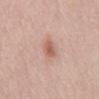workup = catalogued during a skin exam; not biopsied | patient = female, aged around 65 | acquisition = total-body-photography crop, ~15 mm field of view | illumination = white-light | diameter = about 3.5 mm | site = the mid back.